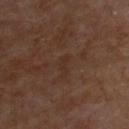Captured during whole-body skin photography for melanoma surveillance; the lesion was not biopsied.
The lesion is located on the chest.
The subject is a male aged around 65.
A 15 mm close-up tile from a total-body photography series done for melanoma screening.
Captured under cross-polarized illumination.
The total-body-photography lesion software estimated a mean CIELAB color near L≈27 a*≈17 b*≈23 and about 4 CIELAB-L* units darker than the surrounding skin. And it measured border irregularity of about 4.5 on a 0–10 scale and radial color variation of about 0. It also reported a classifier nevus-likeness of about 0/100.
Approximately 2.5 mm at its widest.